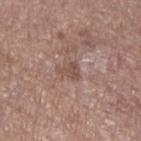Q: Was this lesion biopsied?
A: imaged on a skin check; not biopsied
Q: Where on the body is the lesion?
A: the left lower leg
Q: Lesion size?
A: ≈2.5 mm
Q: How was this image acquired?
A: 15 mm crop, total-body photography
Q: Patient demographics?
A: female, about 65 years old
Q: How was the tile lit?
A: white-light illumination